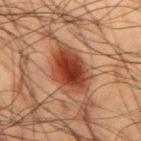This image is a 15 mm lesion crop taken from a total-body photograph.
Approximately 5.5 mm at its widest.
A male patient, aged 58 to 62.
Imaged with cross-polarized lighting.
From the back.